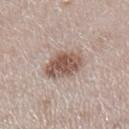No biopsy was performed on this lesion — it was imaged during a full skin examination and was not determined to be concerning. The patient is a female roughly 50 years of age. The total-body-photography lesion software estimated an area of roughly 12 mm², an outline eccentricity of about 0.75 (0 = round, 1 = elongated), and a shape-asymmetry score of about 0.15 (0 = symmetric). The analysis additionally found a mean CIELAB color near L≈54 a*≈17 b*≈24, about 14 CIELAB-L* units darker than the surrounding skin, and a lesion-to-skin contrast of about 9.5 (normalized; higher = more distinct). It also reported border irregularity of about 2 on a 0–10 scale and radial color variation of about 2. And it measured lesion-presence confidence of about 100/100. A lesion tile, about 15 mm wide, cut from a 3D total-body photograph. From the leg. The lesion's longest dimension is about 4.5 mm.